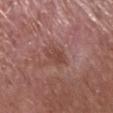{"biopsy_status": "not biopsied; imaged during a skin examination", "patient": {"sex": "male", "age_approx": 65}, "lighting": "white-light", "site": "left forearm", "automated_metrics": {"eccentricity": 0.9, "shape_asymmetry": 0.2}, "image": {"source": "total-body photography crop", "field_of_view_mm": 15}}